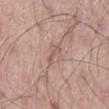<record>
  <biopsy_status>not biopsied; imaged during a skin examination</biopsy_status>
  <image>
    <source>total-body photography crop</source>
    <field_of_view_mm>15</field_of_view_mm>
  </image>
  <lesion_size>
    <long_diameter_mm_approx>3.0</long_diameter_mm_approx>
  </lesion_size>
  <patient>
    <sex>male</sex>
    <age_approx>55</age_approx>
  </patient>
  <site>leg</site>
  <lighting>white-light</lighting>
  <automated_metrics>
    <area_mm2_approx>2.5</area_mm2_approx>
    <eccentricity>0.95</eccentricity>
    <nevus_likeness_0_100>0</nevus_likeness_0_100>
  </automated_metrics>
</record>